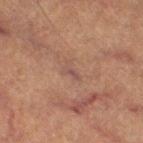Case summary:
• workup: catalogued during a skin exam; not biopsied
• anatomic site: the leg
• patient: female, aged 58 to 62
• image: ~15 mm tile from a whole-body skin photo